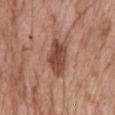The tile uses white-light illumination.
Approximately 4.5 mm at its widest.
A roughly 15 mm field-of-view crop from a total-body skin photograph.
Located on the head or neck.
Automated image analysis of the tile measured an area of roughly 11 mm². It also reported a lesion–skin lightness drop of about 13.
The patient is a female aged 68–72.